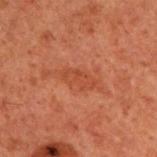{"biopsy_status": "not biopsied; imaged during a skin examination", "image": {"source": "total-body photography crop", "field_of_view_mm": 15}, "automated_metrics": {"area_mm2_approx": 5.0, "eccentricity": 0.9, "shape_asymmetry": 0.25, "cielab_L": 36, "cielab_a": 25, "cielab_b": 29, "vs_skin_darker_L": 5.0, "vs_skin_contrast_norm": 5.0, "border_irregularity_0_10": 2.5, "color_variation_0_10": 2.0, "peripheral_color_asymmetry": 1.0, "nevus_likeness_0_100": 0, "lesion_detection_confidence_0_100": 100}, "lesion_size": {"long_diameter_mm_approx": 3.5}, "patient": {"sex": "male", "age_approx": 60}, "site": "upper back"}